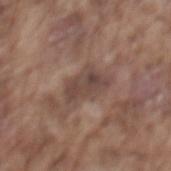Q: Is there a histopathology result?
A: total-body-photography surveillance lesion; no biopsy
Q: How was the tile lit?
A: white-light illumination
Q: Who is the patient?
A: male, aged around 75
Q: What did automated image analysis measure?
A: an area of roughly 7.5 mm², an outline eccentricity of about 0.8 (0 = round, 1 = elongated), and two-axis asymmetry of about 0.35; border irregularity of about 4 on a 0–10 scale, a color-variation rating of about 4.5/10, and radial color variation of about 1.5
Q: What is the lesion's diameter?
A: about 4 mm
Q: Where on the body is the lesion?
A: the mid back
Q: What is the imaging modality?
A: 15 mm crop, total-body photography